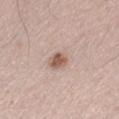Case summary:
• subject: male, roughly 50 years of age
• acquisition: 15 mm crop, total-body photography
• location: the leg
• size: ~4 mm (longest diameter)
• lighting: white-light